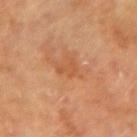notes = total-body-photography surveillance lesion; no biopsy
automated metrics = an average lesion color of about L≈53 a*≈26 b*≈39 (CIELAB), a lesion–skin lightness drop of about 6, and a normalized lesion–skin contrast near 5; an automated nevus-likeness rating near 0 out of 100 and a lesion-detection confidence of about 100/100
subject = female, aged approximately 65
lighting = cross-polarized
location = the arm
image = ~15 mm tile from a whole-body skin photo